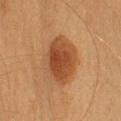Findings:
* tile lighting · cross-polarized illumination
* imaging modality · total-body-photography crop, ~15 mm field of view
* site · the head or neck
* patient · female, aged 18–22